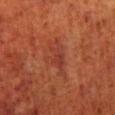<tbp_lesion>
<biopsy_status>not biopsied; imaged during a skin examination</biopsy_status>
<patient>
  <sex>male</sex>
  <age_approx>80</age_approx>
</patient>
<site>right lower leg</site>
<image>
  <source>total-body photography crop</source>
  <field_of_view_mm>15</field_of_view_mm>
</image>
</tbp_lesion>